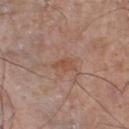Acquisition and patient details: The lesion is located on the leg. Cropped from a total-body skin-imaging series; the visible field is about 15 mm. Automated tile analysis of the lesion measured an area of roughly 3 mm², an outline eccentricity of about 0.85 (0 = round, 1 = elongated), and a shape-asymmetry score of about 0.35 (0 = symmetric). The analysis additionally found a border-irregularity rating of about 3.5/10 and a within-lesion color-variation index near 1.5/10. The analysis additionally found a classifier nevus-likeness of about 0/100 and a detector confidence of about 100 out of 100 that the crop contains a lesion. Measured at roughly 2.5 mm in maximum diameter. A male subject in their 60s.